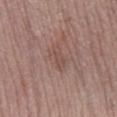Recorded during total-body skin imaging; not selected for excision or biopsy.
The lesion is on the leg.
A 15 mm close-up tile from a total-body photography series done for melanoma screening.
The lesion's longest dimension is about 3.5 mm.
A female subject, approximately 65 years of age.
Captured under white-light illumination.